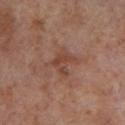Captured during whole-body skin photography for melanoma surveillance; the lesion was not biopsied. The lesion's longest dimension is about 3 mm. A male subject roughly 70 years of age. Automated tile analysis of the lesion measured a lesion area of about 4.5 mm² and an outline eccentricity of about 0.6 (0 = round, 1 = elongated). Captured under cross-polarized illumination. A 15 mm close-up extracted from a 3D total-body photography capture. From the right lower leg.